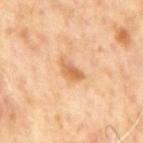Q: Is there a histopathology result?
A: total-body-photography surveillance lesion; no biopsy
Q: Automated lesion metrics?
A: a footprint of about 4 mm², an eccentricity of roughly 0.85, and a symmetry-axis asymmetry near 0.3; a border-irregularity rating of about 3/10 and peripheral color asymmetry of about 0.5
Q: What kind of image is this?
A: total-body-photography crop, ~15 mm field of view
Q: Where on the body is the lesion?
A: the back
Q: What lighting was used for the tile?
A: cross-polarized illumination
Q: Patient demographics?
A: male, approximately 65 years of age